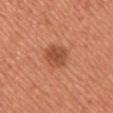Part of a total-body skin-imaging series; this lesion was reviewed on a skin check and was not flagged for biopsy. This image is a 15 mm lesion crop taken from a total-body photograph. From the front of the torso. A female subject, in their 40s.